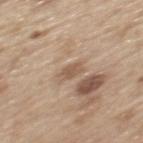The lesion was photographed on a routine skin check and not biopsied; there is no pathology result. A region of skin cropped from a whole-body photographic capture, roughly 15 mm wide. The tile uses white-light illumination. Located on the mid back. A male subject about 70 years old.